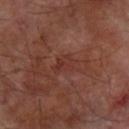Q: Was this lesion biopsied?
A: catalogued during a skin exam; not biopsied
Q: What are the patient's age and sex?
A: male, aged 63 to 67
Q: Lesion size?
A: ~3 mm (longest diameter)
Q: Illumination type?
A: cross-polarized illumination
Q: What is the imaging modality?
A: 15 mm crop, total-body photography
Q: Where on the body is the lesion?
A: the right forearm